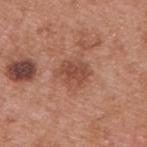Q: Was this lesion biopsied?
A: total-body-photography surveillance lesion; no biopsy
Q: What is the lesion's diameter?
A: ~4 mm (longest diameter)
Q: How was the tile lit?
A: white-light
Q: What are the patient's age and sex?
A: male, aged 53–57
Q: What is the anatomic site?
A: the upper back
Q: What is the imaging modality?
A: total-body-photography crop, ~15 mm field of view